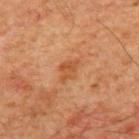<case>
<biopsy_status>not biopsied; imaged during a skin examination</biopsy_status>
<patient>
  <sex>male</sex>
  <age_approx>60</age_approx>
</patient>
<automated_metrics>
  <area_mm2_approx>4.0</area_mm2_approx>
  <eccentricity>0.7</eccentricity>
  <shape_asymmetry>0.3</shape_asymmetry>
  <cielab_L>47</cielab_L>
  <cielab_a>25</cielab_a>
  <cielab_b>37</cielab_b>
  <border_irregularity_0_10>3.0</border_irregularity_0_10>
  <color_variation_0_10>3.0</color_variation_0_10>
  <nevus_likeness_0_100>5</nevus_likeness_0_100>
  <lesion_detection_confidence_0_100>100</lesion_detection_confidence_0_100>
</automated_metrics>
<image>
  <source>total-body photography crop</source>
  <field_of_view_mm>15</field_of_view_mm>
</image>
<site>chest</site>
<lighting>cross-polarized</lighting>
<lesion_size>
  <long_diameter_mm_approx>3.0</long_diameter_mm_approx>
</lesion_size>
</case>